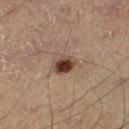follow-up = imaged on a skin check; not biopsied
image-analysis metrics = a normalized border contrast of about 11; a within-lesion color-variation index near 6.5/10 and a peripheral color-asymmetry measure near 1.5; lesion-presence confidence of about 100/100
body site = the left thigh
image = 15 mm crop, total-body photography
tile lighting = cross-polarized
lesion diameter = ~3.5 mm (longest diameter)
subject = male, aged around 60